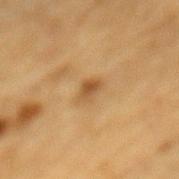<lesion>
  <patient>
    <sex>male</sex>
    <age_approx>85+</age_approx>
  </patient>
  <site>mid back</site>
  <lighting>cross-polarized</lighting>
  <image>
    <source>total-body photography crop</source>
    <field_of_view_mm>15</field_of_view_mm>
  </image>
</lesion>